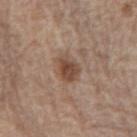workup: catalogued during a skin exam; not biopsied
body site: the left forearm
acquisition: ~15 mm crop, total-body skin-cancer survey
subject: male, roughly 70 years of age